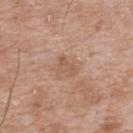Q: Was a biopsy performed?
A: no biopsy performed (imaged during a skin exam)
Q: Where on the body is the lesion?
A: the upper back
Q: Lesion size?
A: ≈2.5 mm
Q: What are the patient's age and sex?
A: male, in their 50s
Q: How was the tile lit?
A: white-light illumination
Q: What kind of image is this?
A: ~15 mm crop, total-body skin-cancer survey